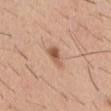<tbp_lesion>
<biopsy_status>not biopsied; imaged during a skin examination</biopsy_status>
<image>
  <source>total-body photography crop</source>
  <field_of_view_mm>15</field_of_view_mm>
</image>
<patient>
  <sex>male</sex>
  <age_approx>40</age_approx>
</patient>
<lesion_size>
  <long_diameter_mm_approx>3.0</long_diameter_mm_approx>
</lesion_size>
<lighting>white-light</lighting>
<site>chest</site>
</tbp_lesion>